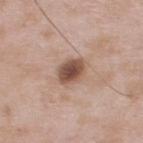notes = catalogued during a skin exam; not biopsied | body site = the back | diameter = ~3 mm (longest diameter) | subject = male, aged 53 to 57 | acquisition = ~15 mm tile from a whole-body skin photo | tile lighting = white-light.